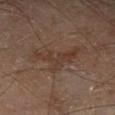follow-up: imaged on a skin check; not biopsied
patient: male, in their 70s
anatomic site: the left lower leg
automated lesion analysis: an average lesion color of about L≈35 a*≈16 b*≈24 (CIELAB), roughly 6 lightness units darker than nearby skin, and a normalized border contrast of about 6; border irregularity of about 9.5 on a 0–10 scale and a peripheral color-asymmetry measure near 1; a nevus-likeness score of about 0/100 and lesion-presence confidence of about 100/100
tile lighting: cross-polarized
image: ~15 mm crop, total-body skin-cancer survey